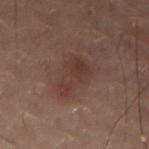Assessment: This lesion was catalogued during total-body skin photography and was not selected for biopsy. Acquisition and patient details: Located on the lower back. A close-up tile cropped from a whole-body skin photograph, about 15 mm across. The subject is a female in their mid-50s.